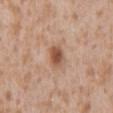Recorded during total-body skin imaging; not selected for excision or biopsy. The lesion is located on the front of the torso. An algorithmic analysis of the crop reported an area of roughly 5.5 mm², a shape eccentricity near 0.65, and a symmetry-axis asymmetry near 0.15. The analysis additionally found roughly 12 lightness units darker than nearby skin and a normalized lesion–skin contrast near 8.5. A 15 mm close-up tile from a total-body photography series done for melanoma screening. The subject is a male aged 43–47. This is a white-light tile.